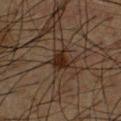This lesion was catalogued during total-body skin photography and was not selected for biopsy.
A roughly 15 mm field-of-view crop from a total-body skin photograph.
This is a cross-polarized tile.
Measured at roughly 3 mm in maximum diameter.
On the chest.
A male patient about 60 years old.